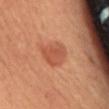Part of a total-body skin-imaging series; this lesion was reviewed on a skin check and was not flagged for biopsy.
The tile uses cross-polarized illumination.
Cropped from a whole-body photographic skin survey; the tile spans about 15 mm.
A female patient.
About 3.5 mm across.
An algorithmic analysis of the crop reported a footprint of about 9 mm², an outline eccentricity of about 0.25 (0 = round, 1 = elongated), and a symmetry-axis asymmetry near 0.25. It also reported a nevus-likeness score of about 90/100 and a lesion-detection confidence of about 100/100.
From the front of the torso.